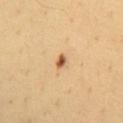workup: no biopsy performed (imaged during a skin exam)
patient: male, approximately 40 years of age
acquisition: total-body-photography crop, ~15 mm field of view
TBP lesion metrics: a border-irregularity index near 3/10, internal color variation of about 1 on a 0–10 scale, and peripheral color asymmetry of about 0; a lesion-detection confidence of about 100/100
tile lighting: cross-polarized
lesion size: ≈2 mm
body site: the chest